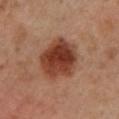* notes — imaged on a skin check; not biopsied
* illumination — cross-polarized
* TBP lesion metrics — internal color variation of about 6 on a 0–10 scale and peripheral color asymmetry of about 2
* patient — male, aged approximately 30
* size — ~5.5 mm (longest diameter)
* acquisition — ~15 mm crop, total-body skin-cancer survey
* anatomic site — the leg